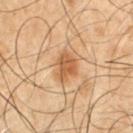A region of skin cropped from a whole-body photographic capture, roughly 15 mm wide. Automated image analysis of the tile measured a footprint of about 7 mm², a shape eccentricity near 0.6, and a shape-asymmetry score of about 0.3 (0 = symmetric). And it measured a lesion color around L≈44 a*≈17 b*≈31 in CIELAB, a lesion–skin lightness drop of about 9, and a lesion-to-skin contrast of about 7.5 (normalized; higher = more distinct). It also reported a border-irregularity index near 3/10, internal color variation of about 3 on a 0–10 scale, and peripheral color asymmetry of about 1. The tile uses cross-polarized illumination. A male patient aged approximately 50. Located on the left upper arm.